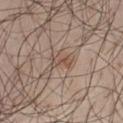The lesion was tiled from a total-body skin photograph and was not biopsied. The lesion is located on the leg. About 3 mm across. An algorithmic analysis of the crop reported a footprint of about 4 mm², a shape eccentricity near 0.85, and two-axis asymmetry of about 0.35. A male patient aged approximately 55. Cropped from a total-body skin-imaging series; the visible field is about 15 mm.